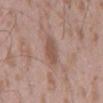Clinical impression:
Imaged during a routine full-body skin examination; the lesion was not biopsied and no histopathology is available.
Acquisition and patient details:
The recorded lesion diameter is about 3.5 mm. The lesion is located on the back. The tile uses white-light illumination. The subject is a male about 50 years old. The lesion-visualizer software estimated a shape eccentricity near 0.8. The analysis additionally found a mean CIELAB color near L≈52 a*≈19 b*≈25, roughly 9 lightness units darker than nearby skin, and a lesion-to-skin contrast of about 6.5 (normalized; higher = more distinct). The software also gave border irregularity of about 2 on a 0–10 scale, a within-lesion color-variation index near 2/10, and peripheral color asymmetry of about 1. Cropped from a whole-body photographic skin survey; the tile spans about 15 mm.